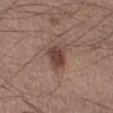Imaged during a routine full-body skin examination; the lesion was not biopsied and no histopathology is available. The patient is a male in their mid-50s. From the right lower leg. Cropped from a whole-body photographic skin survey; the tile spans about 15 mm.